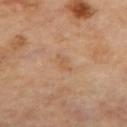Assessment: This lesion was catalogued during total-body skin photography and was not selected for biopsy. Clinical summary: A 15 mm close-up tile from a total-body photography series done for melanoma screening. From the mid back. This is a cross-polarized tile. The recorded lesion diameter is about 2.5 mm.